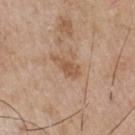No biopsy was performed on this lesion — it was imaged during a full skin examination and was not determined to be concerning.
A 15 mm crop from a total-body photograph taken for skin-cancer surveillance.
Approximately 3.5 mm at its widest.
A male subject, in their mid-60s.
Automated tile analysis of the lesion measured an area of roughly 4 mm², an outline eccentricity of about 0.9 (0 = round, 1 = elongated), and two-axis asymmetry of about 0.4. It also reported a lesion–skin lightness drop of about 9 and a normalized lesion–skin contrast near 7.
Located on the front of the torso.